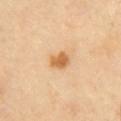Captured during whole-body skin photography for melanoma surveillance; the lesion was not biopsied. The tile uses cross-polarized illumination. The total-body-photography lesion software estimated a lesion area of about 4.5 mm² and a shape eccentricity near 0.7. It also reported an average lesion color of about L≈64 a*≈22 b*≈44 (CIELAB), a lesion–skin lightness drop of about 12, and a normalized lesion–skin contrast near 9. It also reported a border-irregularity index near 2/10, a color-variation rating of about 2.5/10, and radial color variation of about 1. Measured at roughly 3 mm in maximum diameter. From the chest. A male subject aged around 40. A lesion tile, about 15 mm wide, cut from a 3D total-body photograph.